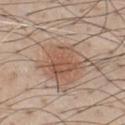Clinical impression:
No biopsy was performed on this lesion — it was imaged during a full skin examination and was not determined to be concerning.
Context:
The lesion is on the chest. About 4 mm across. A male subject, about 40 years old. The total-body-photography lesion software estimated a footprint of about 11 mm², an outline eccentricity of about 0.35 (0 = round, 1 = elongated), and a shape-asymmetry score of about 0.25 (0 = symmetric). The analysis additionally found an average lesion color of about L≈54 a*≈19 b*≈29 (CIELAB). It also reported an automated nevus-likeness rating near 95 out of 100 and a detector confidence of about 100 out of 100 that the crop contains a lesion. A roughly 15 mm field-of-view crop from a total-body skin photograph.